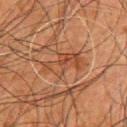follow-up: imaged on a skin check; not biopsied
illumination: cross-polarized illumination
image source: 15 mm crop, total-body photography
lesion diameter: about 4.5 mm
anatomic site: the chest
patient: male, aged around 80